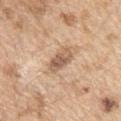Q: Is there a histopathology result?
A: catalogued during a skin exam; not biopsied
Q: Where on the body is the lesion?
A: the left upper arm
Q: How was this image acquired?
A: total-body-photography crop, ~15 mm field of view
Q: What did automated image analysis measure?
A: about 12 CIELAB-L* units darker than the surrounding skin and a lesion-to-skin contrast of about 7.5 (normalized; higher = more distinct); border irregularity of about 2.5 on a 0–10 scale, internal color variation of about 5.5 on a 0–10 scale, and radial color variation of about 2
Q: Who is the patient?
A: male, aged 68 to 72
Q: How large is the lesion?
A: ≈3.5 mm